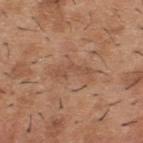{"biopsy_status": "not biopsied; imaged during a skin examination", "site": "upper back", "patient": {"sex": "male", "age_approx": 40}, "image": {"source": "total-body photography crop", "field_of_view_mm": 15}}